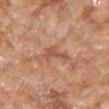Captured during whole-body skin photography for melanoma surveillance; the lesion was not biopsied. About 4 mm across. A female patient aged 73–77. On the right forearm. A lesion tile, about 15 mm wide, cut from a 3D total-body photograph.